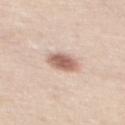| field | value |
|---|---|
| follow-up | imaged on a skin check; not biopsied |
| lesion diameter | about 3.5 mm |
| patient | female, about 45 years old |
| body site | the back |
| image | total-body-photography crop, ~15 mm field of view |